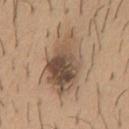Clinical impression:
Imaged during a routine full-body skin examination; the lesion was not biopsied and no histopathology is available.
Image and clinical context:
A lesion tile, about 15 mm wide, cut from a 3D total-body photograph. A male subject, in their 60s. Imaged with white-light lighting. On the mid back.